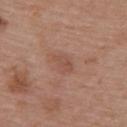Recorded during total-body skin imaging; not selected for excision or biopsy.
Measured at roughly 3 mm in maximum diameter.
Cropped from a whole-body photographic skin survey; the tile spans about 15 mm.
A female subject, aged approximately 60.
The tile uses white-light illumination.
The lesion is located on the back.